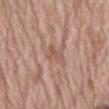Findings:
* notes: catalogued during a skin exam; not biopsied
* image source: ~15 mm tile from a whole-body skin photo
* tile lighting: white-light illumination
* patient: male, aged around 70
* lesion diameter: about 2.5 mm
* location: the abdomen